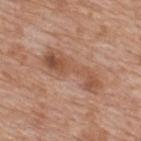Q: Is there a histopathology result?
A: catalogued during a skin exam; not biopsied
Q: Patient demographics?
A: male, aged around 60
Q: How large is the lesion?
A: about 7.5 mm
Q: What lighting was used for the tile?
A: white-light
Q: What kind of image is this?
A: ~15 mm tile from a whole-body skin photo
Q: Lesion location?
A: the upper back